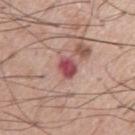Part of a total-body skin-imaging series; this lesion was reviewed on a skin check and was not flagged for biopsy. A roughly 15 mm field-of-view crop from a total-body skin photograph. The lesion's longest dimension is about 2.5 mm. A male patient, in their mid- to late 50s. The total-body-photography lesion software estimated an area of roughly 5 mm², an eccentricity of roughly 0.3, and a shape-asymmetry score of about 0.25 (0 = symmetric). And it measured a lesion–skin lightness drop of about 14 and a normalized lesion–skin contrast near 10.